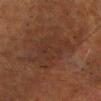No biopsy was performed on this lesion — it was imaged during a full skin examination and was not determined to be concerning. The lesion-visualizer software estimated border irregularity of about 4.5 on a 0–10 scale and a color-variation rating of about 2.5/10. The lesion is located on the head or neck. The subject is a male aged 63 to 67. Longest diameter approximately 6.5 mm. A roughly 15 mm field-of-view crop from a total-body skin photograph.